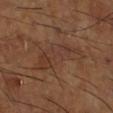The lesion was photographed on a routine skin check and not biopsied; there is no pathology result.